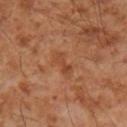Imaged during a routine full-body skin examination; the lesion was not biopsied and no histopathology is available. On the right upper arm. The recorded lesion diameter is about 3.5 mm. The patient is a male aged 53 to 57. The tile uses cross-polarized illumination. Cropped from a whole-body photographic skin survey; the tile spans about 15 mm.